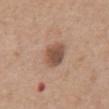biopsy status: no biopsy performed (imaged during a skin exam)
patient: male, roughly 75 years of age
image: ~15 mm crop, total-body skin-cancer survey
anatomic site: the chest